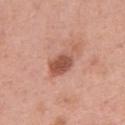No biopsy was performed on this lesion — it was imaged during a full skin examination and was not determined to be concerning. The lesion is on the left upper arm. A close-up tile cropped from a whole-body skin photograph, about 15 mm across. A female subject, approximately 35 years of age.